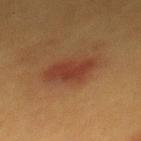This lesion was catalogued during total-body skin photography and was not selected for biopsy.
The recorded lesion diameter is about 4.5 mm.
A female subject, aged around 40.
The lesion is located on the mid back.
Cropped from a whole-body photographic skin survey; the tile spans about 15 mm.